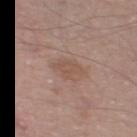workup — catalogued during a skin exam; not biopsied | diameter — ≈2.5 mm | acquisition — total-body-photography crop, ~15 mm field of view | tile lighting — white-light illumination | location — the left thigh | patient — male, aged 53–57.